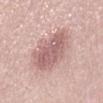Assessment:
Recorded during total-body skin imaging; not selected for excision or biopsy.
Context:
Cropped from a whole-body photographic skin survey; the tile spans about 15 mm. This is a white-light tile. A female subject approximately 40 years of age. The lesion is located on the lower back.